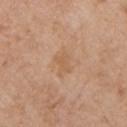Impression:
No biopsy was performed on this lesion — it was imaged during a full skin examination and was not determined to be concerning.
Background:
Measured at roughly 2.5 mm in maximum diameter. A male subject aged 78 to 82. Captured under white-light illumination. A 15 mm close-up tile from a total-body photography series done for melanoma screening. The lesion is located on the chest.